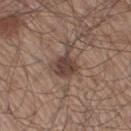Findings:
– biopsy status: imaged on a skin check; not biopsied
– image source: ~15 mm crop, total-body skin-cancer survey
– size: ≈4 mm
– location: the right thigh
– TBP lesion metrics: an eccentricity of roughly 0.55 and two-axis asymmetry of about 0.5; about 11 CIELAB-L* units darker than the surrounding skin and a normalized lesion–skin contrast near 9
– patient: male, aged approximately 60
– lighting: white-light illumination